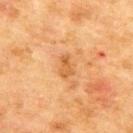notes = total-body-photography surveillance lesion; no biopsy | patient = male, roughly 70 years of age | location = the upper back | lighting = cross-polarized illumination | image source = 15 mm crop, total-body photography | size = ~2.5 mm (longest diameter).